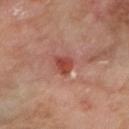This lesion was catalogued during total-body skin photography and was not selected for biopsy. An algorithmic analysis of the crop reported a shape eccentricity near 0.75 and two-axis asymmetry of about 0.3. And it measured a lesion color around L≈46 a*≈30 b*≈29 in CIELAB and a lesion–skin lightness drop of about 10. The software also gave an automated nevus-likeness rating near 80 out of 100 and lesion-presence confidence of about 100/100. Imaged with cross-polarized lighting. A female subject roughly 50 years of age. The lesion is on the right forearm. Approximately 3 mm at its widest. A close-up tile cropped from a whole-body skin photograph, about 15 mm across.